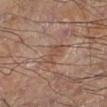– follow-up · imaged on a skin check; not biopsied
– location · the right thigh
– acquisition · ~15 mm crop, total-body skin-cancer survey
– lighting · cross-polarized
– lesion size · ~3 mm (longest diameter)